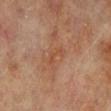Impression: Imaged during a routine full-body skin examination; the lesion was not biopsied and no histopathology is available. Clinical summary: The lesion's longest dimension is about 3 mm. A 15 mm crop from a total-body photograph taken for skin-cancer surveillance. A female subject aged 78–82. The lesion is located on the left lower leg.